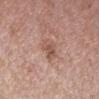Clinical impression:
Recorded during total-body skin imaging; not selected for excision or biopsy.
Image and clinical context:
Approximately 2.5 mm at its widest. A female subject, in their 40s. From the right forearm. Automated tile analysis of the lesion measured a mean CIELAB color near L≈53 a*≈21 b*≈26, roughly 9 lightness units darker than nearby skin, and a normalized lesion–skin contrast near 6.5. The software also gave lesion-presence confidence of about 100/100. Cropped from a total-body skin-imaging series; the visible field is about 15 mm.